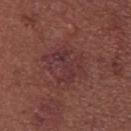No biopsy was performed on this lesion — it was imaged during a full skin examination and was not determined to be concerning.
Located on the upper back.
The lesion's longest dimension is about 5.5 mm.
A female subject roughly 25 years of age.
Automated tile analysis of the lesion measured an eccentricity of roughly 0.6 and a symmetry-axis asymmetry near 0.2. The software also gave a mean CIELAB color near L≈34 a*≈24 b*≈18, roughly 5 lightness units darker than nearby skin, and a normalized lesion–skin contrast near 6.5. And it measured a classifier nevus-likeness of about 0/100.
A close-up tile cropped from a whole-body skin photograph, about 15 mm across.